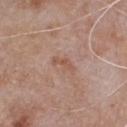Notes:
* workup: imaged on a skin check; not biopsied
* image source: ~15 mm crop, total-body skin-cancer survey
* location: the chest
* automated lesion analysis: an average lesion color of about L≈53 a*≈21 b*≈28 (CIELAB), roughly 7 lightness units darker than nearby skin, and a normalized lesion–skin contrast near 5.5
* subject: male, roughly 80 years of age
* size: ≈3 mm
* tile lighting: white-light